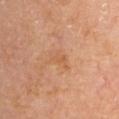Q: Was this lesion biopsied?
A: total-body-photography surveillance lesion; no biopsy
Q: Illumination type?
A: cross-polarized
Q: Automated lesion metrics?
A: a lesion color around L≈56 a*≈22 b*≈36 in CIELAB and a lesion-to-skin contrast of about 4.5 (normalized; higher = more distinct)
Q: What kind of image is this?
A: ~15 mm tile from a whole-body skin photo
Q: Who is the patient?
A: male, aged around 60
Q: Where on the body is the lesion?
A: the left upper arm